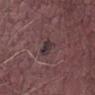Captured during whole-body skin photography for melanoma surveillance; the lesion was not biopsied. The lesion's longest dimension is about 2.5 mm. The total-body-photography lesion software estimated a within-lesion color-variation index near 3.5/10 and peripheral color asymmetry of about 1. Captured under white-light illumination. Cropped from a total-body skin-imaging series; the visible field is about 15 mm. Located on the right thigh. A male patient, in their mid- to late 70s.